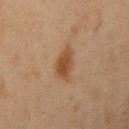{
  "biopsy_status": "not biopsied; imaged during a skin examination",
  "lesion_size": {
    "long_diameter_mm_approx": 3.5
  },
  "patient": {
    "sex": "female",
    "age_approx": 45
  },
  "site": "right upper arm",
  "lighting": "cross-polarized",
  "image": {
    "source": "total-body photography crop",
    "field_of_view_mm": 15
  }
}